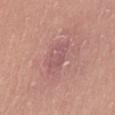anatomic site: the left thigh | automated lesion analysis: a border-irregularity rating of about 3/10, a color-variation rating of about 3/10, and a peripheral color-asymmetry measure near 1; a classifier nevus-likeness of about 0/100 and a detector confidence of about 95 out of 100 that the crop contains a lesion | acquisition: 15 mm crop, total-body photography | lighting: white-light | patient: female, roughly 40 years of age.